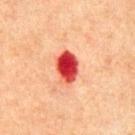Part of a total-body skin-imaging series; this lesion was reviewed on a skin check and was not flagged for biopsy. Cropped from a whole-body photographic skin survey; the tile spans about 15 mm. A male patient, aged around 65. From the chest. Imaged with cross-polarized lighting. Automated image analysis of the tile measured a footprint of about 8 mm², an outline eccentricity of about 0.75 (0 = round, 1 = elongated), and a symmetry-axis asymmetry near 0.15. And it measured a mean CIELAB color near L≈45 a*≈44 b*≈34 and roughly 21 lightness units darker than nearby skin. It also reported a detector confidence of about 100 out of 100 that the crop contains a lesion. Measured at roughly 4 mm in maximum diameter.